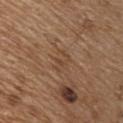Part of a total-body skin-imaging series; this lesion was reviewed on a skin check and was not flagged for biopsy. A male patient, approximately 70 years of age. A region of skin cropped from a whole-body photographic capture, roughly 15 mm wide. Located on the chest. The tile uses white-light illumination.